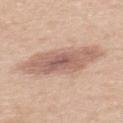biopsy status = catalogued during a skin exam; not biopsied | patient = male, aged 28 to 32 | body site = the back | image source = ~15 mm tile from a whole-body skin photo | lesion diameter = ~8.5 mm (longest diameter) | lighting = white-light.